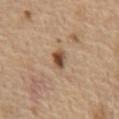Clinical impression: Captured during whole-body skin photography for melanoma surveillance; the lesion was not biopsied. Acquisition and patient details: The total-body-photography lesion software estimated a lesion area of about 3.5 mm², an outline eccentricity of about 0.8 (0 = round, 1 = elongated), and a symmetry-axis asymmetry near 0.2. And it measured border irregularity of about 2 on a 0–10 scale, a color-variation rating of about 3.5/10, and a peripheral color-asymmetry measure near 1. The analysis additionally found a classifier nevus-likeness of about 90/100 and a lesion-detection confidence of about 100/100. Measured at roughly 2.5 mm in maximum diameter. Located on the chest. A 15 mm close-up extracted from a 3D total-body photography capture. A male patient approximately 70 years of age.